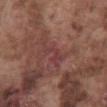Findings:
* follow-up — imaged on a skin check; not biopsied
* TBP lesion metrics — an average lesion color of about L≈39 a*≈25 b*≈21 (CIELAB), roughly 5 lightness units darker than nearby skin, and a lesion-to-skin contrast of about 4.5 (normalized; higher = more distinct); a border-irregularity index near 6.5/10, a color-variation rating of about 0/10, and a peripheral color-asymmetry measure near 0
* illumination — white-light
* lesion size — about 3 mm
* body site — the abdomen
* patient — male, approximately 75 years of age
* image source — 15 mm crop, total-body photography